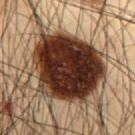{"biopsy_status": "not biopsied; imaged during a skin examination", "automated_metrics": {"area_mm2_approx": 48.0, "eccentricity": 0.5, "shape_asymmetry": 0.15, "border_irregularity_0_10": 2.0, "color_variation_0_10": 6.5, "peripheral_color_asymmetry": 2.0}, "patient": {"sex": "male", "age_approx": 55}, "lesion_size": {"long_diameter_mm_approx": 8.5}, "site": "abdomen", "lighting": "cross-polarized", "image": {"source": "total-body photography crop", "field_of_view_mm": 15}}